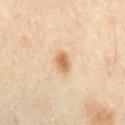follow-up: no biopsy performed (imaged during a skin exam)
location: the front of the torso
subject: male, in their mid-50s
image: total-body-photography crop, ~15 mm field of view
diameter: ~3 mm (longest diameter)
tile lighting: cross-polarized illumination